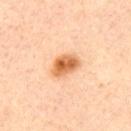biopsy status=no biopsy performed (imaged during a skin exam)
acquisition=total-body-photography crop, ~15 mm field of view
location=the upper back
lesion diameter=about 3.5 mm
patient=male, aged approximately 35
lighting=cross-polarized